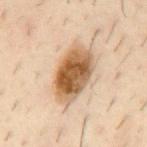No biopsy was performed on this lesion — it was imaged during a full skin examination and was not determined to be concerning.